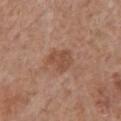Recorded during total-body skin imaging; not selected for excision or biopsy. The patient is a female roughly 70 years of age. The tile uses white-light illumination. A 15 mm close-up extracted from a 3D total-body photography capture. The lesion is on the chest. The lesion's longest dimension is about 3 mm.